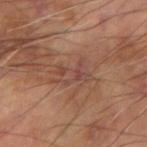Part of a total-body skin-imaging series; this lesion was reviewed on a skin check and was not flagged for biopsy. The total-body-photography lesion software estimated a footprint of about 6.5 mm², an eccentricity of roughly 0.8, and a symmetry-axis asymmetry near 0.4. And it measured a border-irregularity index near 5.5/10, internal color variation of about 5.5 on a 0–10 scale, and peripheral color asymmetry of about 1.5. Located on the left arm. Longest diameter approximately 4 mm. A male subject approximately 60 years of age. The tile uses cross-polarized illumination. A lesion tile, about 15 mm wide, cut from a 3D total-body photograph.